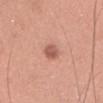Impression:
Part of a total-body skin-imaging series; this lesion was reviewed on a skin check and was not flagged for biopsy.
Clinical summary:
This is a white-light tile. Located on the head or neck. A 15 mm close-up tile from a total-body photography series done for melanoma screening. Measured at roughly 2.5 mm in maximum diameter. An algorithmic analysis of the crop reported an area of roughly 4 mm², an eccentricity of roughly 0.6, and a shape-asymmetry score of about 0.15 (0 = symmetric). And it measured border irregularity of about 1 on a 0–10 scale and peripheral color asymmetry of about 1. A female subject, aged 33–37.